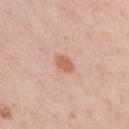Imaged during a routine full-body skin examination; the lesion was not biopsied and no histopathology is available.
A male subject, aged around 60.
From the chest.
Cropped from a total-body skin-imaging series; the visible field is about 15 mm.
Longest diameter approximately 2.5 mm.
The tile uses white-light illumination.
The total-body-photography lesion software estimated an average lesion color of about L≈62 a*≈22 b*≈32 (CIELAB) and about 10 CIELAB-L* units darker than the surrounding skin. The analysis additionally found a nevus-likeness score of about 30/100 and a detector confidence of about 100 out of 100 that the crop contains a lesion.